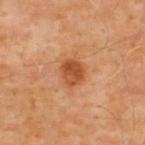Imaged during a routine full-body skin examination; the lesion was not biopsied and no histopathology is available. A 15 mm close-up extracted from a 3D total-body photography capture. The lesion-visualizer software estimated a footprint of about 6 mm², a shape eccentricity near 0.55, and a shape-asymmetry score of about 0.2 (0 = symmetric). And it measured a lesion-detection confidence of about 100/100. Captured under cross-polarized illumination. Longest diameter approximately 3 mm. The lesion is located on the back. The subject is a male aged 53–57.